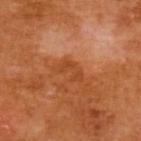Assessment: The lesion was photographed on a routine skin check and not biopsied; there is no pathology result. Clinical summary: This is a cross-polarized tile. Approximately 3.5 mm at its widest. The lesion is on the upper back. Automated image analysis of the tile measured a lesion area of about 5 mm² and an outline eccentricity of about 0.85 (0 = round, 1 = elongated). The software also gave an average lesion color of about L≈47 a*≈30 b*≈42 (CIELAB), about 7 CIELAB-L* units darker than the surrounding skin, and a lesion-to-skin contrast of about 6 (normalized; higher = more distinct). A close-up tile cropped from a whole-body skin photograph, about 15 mm across. A female patient aged around 55.